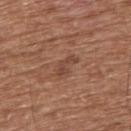Clinical impression: This lesion was catalogued during total-body skin photography and was not selected for biopsy. Context: Longest diameter approximately 3 mm. Imaged with white-light lighting. On the upper back. A male subject, aged 73–77. This image is a 15 mm lesion crop taken from a total-body photograph.